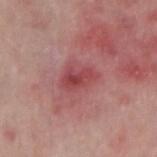Impression:
Captured during whole-body skin photography for melanoma surveillance; the lesion was not biopsied.
Image and clinical context:
From the right forearm. The tile uses white-light illumination. A male patient in their mid-70s. A 15 mm close-up tile from a total-body photography series done for melanoma screening.